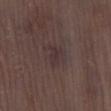Findings:
– biopsy status: total-body-photography surveillance lesion; no biopsy
– diameter: ~2.5 mm (longest diameter)
– site: the leg
– patient: male, aged 68 to 72
– tile lighting: white-light
– image: 15 mm crop, total-body photography